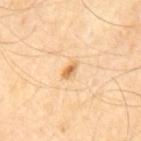Clinical impression:
Captured during whole-body skin photography for melanoma surveillance; the lesion was not biopsied.
Acquisition and patient details:
Approximately 2.5 mm at its widest. A male patient, aged 53 to 57. The lesion is located on the mid back. A 15 mm crop from a total-body photograph taken for skin-cancer surveillance. The total-body-photography lesion software estimated a lesion color around L≈68 a*≈21 b*≈43 in CIELAB and a lesion–skin lightness drop of about 11. This is a cross-polarized tile.